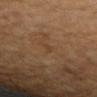No biopsy was performed on this lesion — it was imaged during a full skin examination and was not determined to be concerning. The lesion's longest dimension is about 1 mm. A female subject, roughly 65 years of age. Captured under cross-polarized illumination. Cropped from a whole-body photographic skin survey; the tile spans about 15 mm. The lesion-visualizer software estimated a nevus-likeness score of about 0/100 and lesion-presence confidence of about 100/100. On the arm.